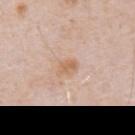Imaged during a routine full-body skin examination; the lesion was not biopsied and no histopathology is available. This image is a 15 mm lesion crop taken from a total-body photograph. Measured at roughly 2.5 mm in maximum diameter. The lesion is located on the chest. Captured under white-light illumination. A male subject, approximately 80 years of age.